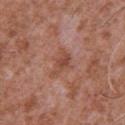Q: Lesion size?
A: ~3.5 mm (longest diameter)
Q: What did automated image analysis measure?
A: an area of roughly 4.5 mm², an outline eccentricity of about 0.85 (0 = round, 1 = elongated), and two-axis asymmetry of about 0.45
Q: What are the patient's age and sex?
A: male, aged around 45
Q: Lesion location?
A: the chest
Q: How was this image acquired?
A: total-body-photography crop, ~15 mm field of view
Q: How was the tile lit?
A: white-light illumination